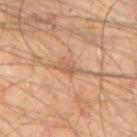notes — total-body-photography surveillance lesion; no biopsy | body site — the mid back | patient — male, aged around 65 | size — about 3 mm | acquisition — ~15 mm tile from a whole-body skin photo | lighting — cross-polarized illumination.